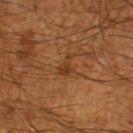This lesion was catalogued during total-body skin photography and was not selected for biopsy. Located on the left thigh. A male subject, aged 58–62. Imaged with cross-polarized lighting. This image is a 15 mm lesion crop taken from a total-body photograph.